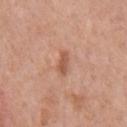biopsy_status: not biopsied; imaged during a skin examination
patient:
  sex: male
  age_approx: 70
site: chest
lighting: white-light
automated_metrics:
  border_irregularity_0_10: 3.0
  color_variation_0_10: 1.0
  peripheral_color_asymmetry: 0.0
image:
  source: total-body photography crop
  field_of_view_mm: 15